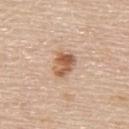Notes:
- biopsy status · total-body-photography surveillance lesion; no biopsy
- site · the upper back
- image-analysis metrics · an area of roughly 6.5 mm² and a symmetry-axis asymmetry near 0.25; a border-irregularity rating of about 2.5/10; a classifier nevus-likeness of about 90/100 and lesion-presence confidence of about 100/100
- subject · male, roughly 60 years of age
- tile lighting · white-light
- image source · total-body-photography crop, ~15 mm field of view